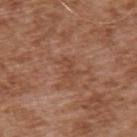Assessment: This lesion was catalogued during total-body skin photography and was not selected for biopsy. Context: The lesion is located on the upper back. A male patient, in their mid- to late 70s. Cropped from a total-body skin-imaging series; the visible field is about 15 mm.